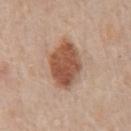The lesion is located on the chest. A male subject, about 80 years old. A close-up tile cropped from a whole-body skin photograph, about 15 mm across. Longest diameter approximately 6.5 mm. Imaged with white-light lighting.